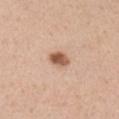Case summary:
– follow-up — catalogued during a skin exam; not biopsied
– diameter — ~3 mm (longest diameter)
– patient — male, aged approximately 40
– automated metrics — an area of roughly 4.5 mm², an outline eccentricity of about 0.7 (0 = round, 1 = elongated), and a shape-asymmetry score of about 0.2 (0 = symmetric); internal color variation of about 5 on a 0–10 scale and radial color variation of about 2
– site — the right upper arm
– imaging modality — total-body-photography crop, ~15 mm field of view
– illumination — white-light illumination